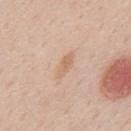Part of a total-body skin-imaging series; this lesion was reviewed on a skin check and was not flagged for biopsy.
Longest diameter approximately 3.5 mm.
A region of skin cropped from a whole-body photographic capture, roughly 15 mm wide.
Located on the mid back.
A male subject aged 58 to 62.